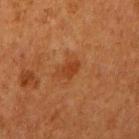Captured during whole-body skin photography for melanoma surveillance; the lesion was not biopsied. Automated image analysis of the tile measured a symmetry-axis asymmetry near 0.35. The analysis additionally found a classifier nevus-likeness of about 35/100 and a detector confidence of about 100 out of 100 that the crop contains a lesion. A female subject about 50 years old. The lesion is on the right upper arm. Approximately 3 mm at its widest. Imaged with cross-polarized lighting. A 15 mm crop from a total-body photograph taken for skin-cancer surveillance.